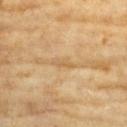The lesion was photographed on a routine skin check and not biopsied; there is no pathology result. A female subject aged approximately 75. Located on the chest. A close-up tile cropped from a whole-body skin photograph, about 15 mm across.